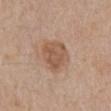patient:
  sex: male
  age_approx: 80
image:
  source: total-body photography crop
  field_of_view_mm: 15
site: front of the torso